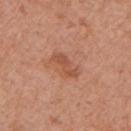Captured during whole-body skin photography for melanoma surveillance; the lesion was not biopsied.
A female subject aged approximately 65.
A lesion tile, about 15 mm wide, cut from a 3D total-body photograph.
Located on the left upper arm.